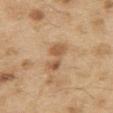This lesion was catalogued during total-body skin photography and was not selected for biopsy. From the upper back. Automated tile analysis of the lesion measured a lesion color around L≈57 a*≈19 b*≈36 in CIELAB and about 10 CIELAB-L* units darker than the surrounding skin. The analysis additionally found internal color variation of about 3.5 on a 0–10 scale and radial color variation of about 1. It also reported a lesion-detection confidence of about 100/100. The subject is a male approximately 70 years of age. Cropped from a whole-body photographic skin survey; the tile spans about 15 mm.